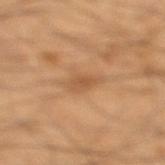Impression: The lesion was photographed on a routine skin check and not biopsied; there is no pathology result. Acquisition and patient details: A 15 mm close-up extracted from a 3D total-body photography capture. The subject is a male in their 40s. Located on the right lower leg. This is a cross-polarized tile. The recorded lesion diameter is about 4.5 mm. An algorithmic analysis of the crop reported a border-irregularity rating of about 4.5/10, internal color variation of about 1 on a 0–10 scale, and radial color variation of about 0.5.